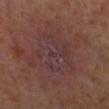  biopsy_status: not biopsied; imaged during a skin examination
  patient:
    sex: female
  image:
    source: total-body photography crop
    field_of_view_mm: 15
  site: left lower leg
  lesion_size:
    long_diameter_mm_approx: 5.5
  automated_metrics:
    area_mm2_approx: 23.0
    eccentricity: 0.4
    shape_asymmetry: 0.3
    cielab_L: 36
    cielab_a: 19
    cielab_b: 16
    vs_skin_darker_L: 4.0
    vs_skin_contrast_norm: 6.5
    border_irregularity_0_10: 4.0
    color_variation_0_10: 4.0
    peripheral_color_asymmetry: 1.5